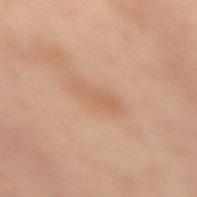Assessment:
Imaged during a routine full-body skin examination; the lesion was not biopsied and no histopathology is available.
Background:
Automated tile analysis of the lesion measured an average lesion color of about L≈50 a*≈17 b*≈28 (CIELAB), about 5 CIELAB-L* units darker than the surrounding skin, and a normalized border contrast of about 4.5. The lesion is located on the back. Cropped from a whole-body photographic skin survey; the tile spans about 15 mm. A female subject aged around 55. Captured under cross-polarized illumination. Measured at roughly 4 mm in maximum diameter.